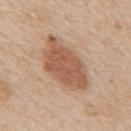No biopsy was performed on this lesion — it was imaged during a full skin examination and was not determined to be concerning. The lesion is located on the mid back. An algorithmic analysis of the crop reported a border-irregularity rating of about 2.5/10, a within-lesion color-variation index near 3/10, and radial color variation of about 1. The software also gave an automated nevus-likeness rating near 90 out of 100 and a detector confidence of about 100 out of 100 that the crop contains a lesion. A male subject aged 58 to 62. Cropped from a whole-body photographic skin survey; the tile spans about 15 mm. The lesion's longest dimension is about 7.5 mm. Captured under white-light illumination.